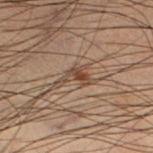Imaged during a routine full-body skin examination; the lesion was not biopsied and no histopathology is available. Located on the left lower leg. Approximately 4.5 mm at its widest. A male subject aged approximately 55. This image is a 15 mm lesion crop taken from a total-body photograph.